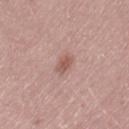Case summary:
* patient · female, approximately 50 years of age
* location · the left thigh
* illumination · white-light
* acquisition · total-body-photography crop, ~15 mm field of view
* size · ~2.5 mm (longest diameter)